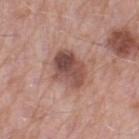{
  "biopsy_status": "not biopsied; imaged during a skin examination",
  "patient": {
    "sex": "male",
    "age_approx": 55
  },
  "image": {
    "source": "total-body photography crop",
    "field_of_view_mm": 15
  },
  "site": "right thigh",
  "lesion_size": {
    "long_diameter_mm_approx": 4.5
  }
}